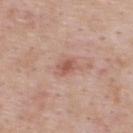This lesion was catalogued during total-body skin photography and was not selected for biopsy. Imaged with white-light lighting. Automated tile analysis of the lesion measured a nevus-likeness score of about 15/100 and lesion-presence confidence of about 100/100. A lesion tile, about 15 mm wide, cut from a 3D total-body photograph. From the upper back. A male patient aged around 55.